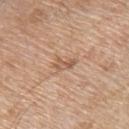workup = catalogued during a skin exam; not biopsied
image = total-body-photography crop, ~15 mm field of view
site = the left upper arm
illumination = white-light
subject = male, roughly 65 years of age
size = about 2.5 mm